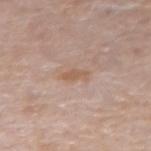<lesion>
<biopsy_status>not biopsied; imaged during a skin examination</biopsy_status>
<lighting>white-light</lighting>
<patient>
  <sex>female</sex>
  <age_approx>65</age_approx>
</patient>
<automated_metrics>
  <area_mm2_approx>3.5</area_mm2_approx>
  <cielab_L>58</cielab_L>
  <cielab_a>18</cielab_a>
  <cielab_b>29</cielab_b>
  <vs_skin_darker_L>7.0</vs_skin_darker_L>
  <vs_skin_contrast_norm>6.0</vs_skin_contrast_norm>
  <nevus_likeness_0_100>0</nevus_likeness_0_100>
  <lesion_detection_confidence_0_100>100</lesion_detection_confidence_0_100>
</automated_metrics>
<site>left forearm</site>
<image>
  <source>total-body photography crop</source>
  <field_of_view_mm>15</field_of_view_mm>
</image>
</lesion>